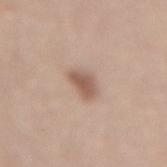The lesion was photographed on a routine skin check and not biopsied; there is no pathology result. The patient is a male aged 63 to 67. Imaged with white-light lighting. Longest diameter approximately 2.5 mm. A close-up tile cropped from a whole-body skin photograph, about 15 mm across. From the lower back. The total-body-photography lesion software estimated two-axis asymmetry of about 0.3. The software also gave roughly 12 lightness units darker than nearby skin and a normalized border contrast of about 8. The software also gave a border-irregularity rating of about 2.5/10, a color-variation rating of about 2.5/10, and peripheral color asymmetry of about 0.5.